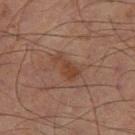<tbp_lesion>
<biopsy_status>not biopsied; imaged during a skin examination</biopsy_status>
<automated_metrics>
  <cielab_L>33</cielab_L>
  <cielab_a>17</cielab_a>
  <cielab_b>24</cielab_b>
  <vs_skin_contrast_norm>7.0</vs_skin_contrast_norm>
  <border_irregularity_0_10>6.0</border_irregularity_0_10>
  <peripheral_color_asymmetry>0.5</peripheral_color_asymmetry>
  <nevus_likeness_0_100>0</nevus_likeness_0_100>
  <lesion_detection_confidence_0_100>100</lesion_detection_confidence_0_100>
</automated_metrics>
<lighting>cross-polarized</lighting>
<patient>
  <sex>male</sex>
  <age_approx>70</age_approx>
</patient>
<lesion_size>
  <long_diameter_mm_approx>3.5</long_diameter_mm_approx>
</lesion_size>
<image>
  <source>total-body photography crop</source>
  <field_of_view_mm>15</field_of_view_mm>
</image>
<site>right thigh</site>
</tbp_lesion>